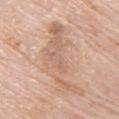From the upper back.
Cropped from a total-body skin-imaging series; the visible field is about 15 mm.
A female patient about 65 years old.